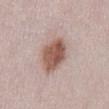Q: Is there a histopathology result?
A: catalogued during a skin exam; not biopsied
Q: What is the lesion's diameter?
A: ~5 mm (longest diameter)
Q: How was this image acquired?
A: ~15 mm tile from a whole-body skin photo
Q: Patient demographics?
A: female, aged 48–52
Q: Automated lesion metrics?
A: border irregularity of about 1.5 on a 0–10 scale, a color-variation rating of about 5/10, and a peripheral color-asymmetry measure near 1.5; a nevus-likeness score of about 100/100
Q: Lesion location?
A: the abdomen